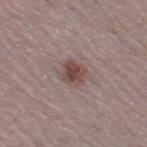notes: imaged on a skin check; not biopsied
patient: male, about 65 years old
acquisition: 15 mm crop, total-body photography
site: the leg
lesion size: about 3 mm
illumination: white-light